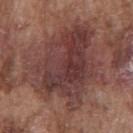This is a white-light tile. A lesion tile, about 15 mm wide, cut from a 3D total-body photograph. The lesion is located on the front of the torso. A male subject about 75 years old. Longest diameter approximately 10.5 mm. The lesion-visualizer software estimated an average lesion color of about L≈39 a*≈21 b*≈21 (CIELAB) and a lesion-to-skin contrast of about 9 (normalized; higher = more distinct). The software also gave a border-irregularity index near 5.5/10, internal color variation of about 7 on a 0–10 scale, and a peripheral color-asymmetry measure near 2.5. It also reported a nevus-likeness score of about 0/100 and a detector confidence of about 80 out of 100 that the crop contains a lesion.